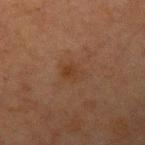{
  "biopsy_status": "not biopsied; imaged during a skin examination",
  "lesion_size": {
    "long_diameter_mm_approx": 3.0
  },
  "lighting": "cross-polarized",
  "site": "right upper arm",
  "image": {
    "source": "total-body photography crop",
    "field_of_view_mm": 15
  },
  "patient": {
    "sex": "male",
    "age_approx": 65
  }
}